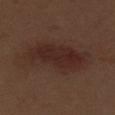notes: total-body-photography surveillance lesion; no biopsy
imaging modality: ~15 mm tile from a whole-body skin photo
subject: male, aged 68–72
body site: the right thigh